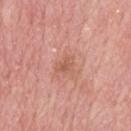biopsy_status: not biopsied; imaged during a skin examination
site: upper back
lesion_size:
  long_diameter_mm_approx: 2.5
automated_metrics:
  cielab_L: 58
  cielab_a: 25
  cielab_b: 30
  vs_skin_contrast_norm: 5.5
  border_irregularity_0_10: 3.0
  color_variation_0_10: 1.5
  peripheral_color_asymmetry: 0.5
  nevus_likeness_0_100: 0
  lesion_detection_confidence_0_100: 100
lighting: white-light
image:
  source: total-body photography crop
  field_of_view_mm: 15
patient:
  sex: male
  age_approx: 50